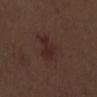Imaged during a routine full-body skin examination; the lesion was not biopsied and no histopathology is available. A 15 mm close-up extracted from a 3D total-body photography capture. The lesion-visualizer software estimated a lesion color around L≈25 a*≈17 b*≈19 in CIELAB and a lesion-to-skin contrast of about 6.5 (normalized; higher = more distinct). And it measured a border-irregularity index near 5/10, a color-variation rating of about 2/10, and a peripheral color-asymmetry measure near 0.5. The software also gave an automated nevus-likeness rating near 25 out of 100 and lesion-presence confidence of about 100/100. Located on the arm. A male subject, about 70 years old. Imaged with white-light lighting.